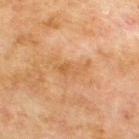workup: no biopsy performed (imaged during a skin exam)
image source: total-body-photography crop, ~15 mm field of view
TBP lesion metrics: an area of roughly 4 mm² and two-axis asymmetry of about 0.5; a peripheral color-asymmetry measure near 0; lesion-presence confidence of about 100/100
patient: male, aged 68 to 72
body site: the upper back